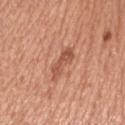Impression: The lesion was tiled from a total-body skin photograph and was not biopsied. Background: Imaged with white-light lighting. The subject is a female roughly 50 years of age. On the right upper arm. A 15 mm close-up extracted from a 3D total-body photography capture.